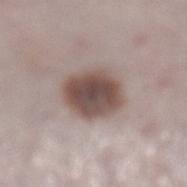Q: Who is the patient?
A: female, about 50 years old
Q: How large is the lesion?
A: ≈5 mm
Q: What is the imaging modality?
A: ~15 mm crop, total-body skin-cancer survey
Q: Automated lesion metrics?
A: border irregularity of about 1.5 on a 0–10 scale, internal color variation of about 5 on a 0–10 scale, and a peripheral color-asymmetry measure near 1.5; a classifier nevus-likeness of about 60/100
Q: Where on the body is the lesion?
A: the left lower leg
Q: Illumination type?
A: white-light